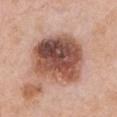Imaged during a routine full-body skin examination; the lesion was not biopsied and no histopathology is available. Automated tile analysis of the lesion measured an area of roughly 35 mm², an outline eccentricity of about 0.4 (0 = round, 1 = elongated), and a symmetry-axis asymmetry near 0.1. The analysis additionally found an average lesion color of about L≈51 a*≈22 b*≈27 (CIELAB), a lesion–skin lightness drop of about 18, and a normalized lesion–skin contrast near 11.5. And it measured a detector confidence of about 100 out of 100 that the crop contains a lesion. Captured under white-light illumination. A female patient roughly 60 years of age. The lesion is on the front of the torso. This image is a 15 mm lesion crop taken from a total-body photograph. About 7 mm across.